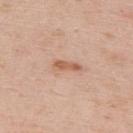  biopsy_status: not biopsied; imaged during a skin examination
  image:
    source: total-body photography crop
    field_of_view_mm: 15
  site: upper back
  automated_metrics:
    area_mm2_approx: 3.5
    eccentricity: 0.9
    border_irregularity_0_10: 3.0
    color_variation_0_10: 1.0
  patient:
    sex: male
    age_approx: 40
  lesion_size:
    long_diameter_mm_approx: 3.5